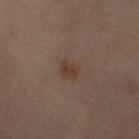Clinical impression:
No biopsy was performed on this lesion — it was imaged during a full skin examination and was not determined to be concerning.
Clinical summary:
The lesion is on the left leg. Captured under cross-polarized illumination. A close-up tile cropped from a whole-body skin photograph, about 15 mm across. Approximately 2.5 mm at its widest. The lesion-visualizer software estimated border irregularity of about 3 on a 0–10 scale and peripheral color asymmetry of about 0.5. The analysis additionally found an automated nevus-likeness rating near 70 out of 100 and lesion-presence confidence of about 100/100. A female patient, aged 58–62.